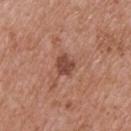Clinical impression: Part of a total-body skin-imaging series; this lesion was reviewed on a skin check and was not flagged for biopsy. Acquisition and patient details: Cropped from a whole-body photographic skin survey; the tile spans about 15 mm. This is a white-light tile. The subject is a male aged approximately 55. Located on the upper back.